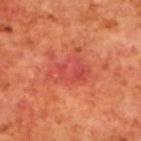follow-up — total-body-photography surveillance lesion; no biopsy | anatomic site — the upper back | image — ~15 mm crop, total-body skin-cancer survey | subject — male, about 70 years old | lighting — cross-polarized.